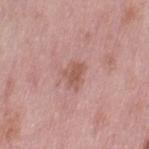No biopsy was performed on this lesion — it was imaged during a full skin examination and was not determined to be concerning. Automated tile analysis of the lesion measured a lesion area of about 6 mm² and a shape-asymmetry score of about 0.3 (0 = symmetric). A female subject, roughly 50 years of age. Cropped from a whole-body photographic skin survey; the tile spans about 15 mm. The lesion is on the leg. Measured at roughly 3 mm in maximum diameter.